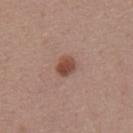Part of a total-body skin-imaging series; this lesion was reviewed on a skin check and was not flagged for biopsy.
The lesion-visualizer software estimated an outline eccentricity of about 0.7 (0 = round, 1 = elongated) and a shape-asymmetry score of about 0.2 (0 = symmetric). It also reported roughly 12 lightness units darker than nearby skin and a normalized border contrast of about 9.5. The software also gave an automated nevus-likeness rating near 100 out of 100.
On the back.
A 15 mm close-up tile from a total-body photography series done for melanoma screening.
The tile uses white-light illumination.
The subject is a male in their mid- to late 50s.